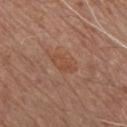Q: Was this lesion biopsied?
A: catalogued during a skin exam; not biopsied
Q: How was the tile lit?
A: white-light
Q: What is the anatomic site?
A: the chest
Q: What is the lesion's diameter?
A: ~3.5 mm (longest diameter)
Q: How was this image acquired?
A: ~15 mm tile from a whole-body skin photo
Q: What are the patient's age and sex?
A: male, aged approximately 55